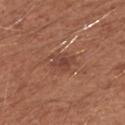Recorded during total-body skin imaging; not selected for excision or biopsy. An algorithmic analysis of the crop reported a footprint of about 5 mm² and a shape eccentricity near 0.6. The software also gave a color-variation rating of about 3.5/10 and radial color variation of about 1. The analysis additionally found an automated nevus-likeness rating near 0 out of 100 and a lesion-detection confidence of about 100/100. This is a white-light tile. From the arm. A female subject, aged 38 to 42. Longest diameter approximately 3 mm. Cropped from a total-body skin-imaging series; the visible field is about 15 mm.